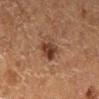Impression:
The lesion was photographed on a routine skin check and not biopsied; there is no pathology result.
Background:
A lesion tile, about 15 mm wide, cut from a 3D total-body photograph. Imaged with cross-polarized lighting. Approximately 3.5 mm at its widest. From the left lower leg. The total-body-photography lesion software estimated an average lesion color of about L≈31 a*≈18 b*≈24 (CIELAB), about 10 CIELAB-L* units darker than the surrounding skin, and a normalized border contrast of about 10. The software also gave a classifier nevus-likeness of about 80/100. A female subject, about 50 years old.